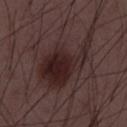notes: no biopsy performed (imaged during a skin exam) | imaging modality: ~15 mm crop, total-body skin-cancer survey | patient: male, approximately 50 years of age.